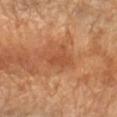Assessment:
Recorded during total-body skin imaging; not selected for excision or biopsy.